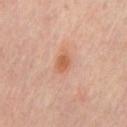Captured during whole-body skin photography for melanoma surveillance; the lesion was not biopsied. Captured under cross-polarized illumination. Measured at roughly 2.5 mm in maximum diameter. A lesion tile, about 15 mm wide, cut from a 3D total-body photograph. Automated image analysis of the tile measured border irregularity of about 1.5 on a 0–10 scale and a color-variation rating of about 2/10. A male subject approximately 65 years of age. From the chest.